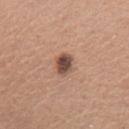The lesion was photographed on a routine skin check and not biopsied; there is no pathology result. A female patient, aged 53–57. The tile uses white-light illumination. The lesion is on the chest. A close-up tile cropped from a whole-body skin photograph, about 15 mm across. Automated tile analysis of the lesion measured a lesion area of about 5 mm², a shape eccentricity near 0.7, and two-axis asymmetry of about 0.15. The analysis additionally found an automated nevus-likeness rating near 85 out of 100 and lesion-presence confidence of about 100/100. Approximately 3 mm at its widest.